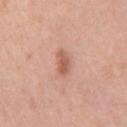  biopsy_status: not biopsied; imaged during a skin examination
  image:
    source: total-body photography crop
    field_of_view_mm: 15
  site: right upper arm
  lesion_size:
    long_diameter_mm_approx: 3.0
  patient:
    sex: male
    age_approx: 50
  lighting: white-light
  automated_metrics:
    cielab_L: 59
    cielab_a: 24
    cielab_b: 30
    vs_skin_darker_L: 10.0
    border_irregularity_0_10: 2.5
    color_variation_0_10: 3.0
    peripheral_color_asymmetry: 1.0
    lesion_detection_confidence_0_100: 100